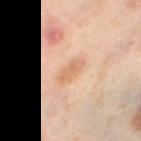Impression: Recorded during total-body skin imaging; not selected for excision or biopsy. Acquisition and patient details: Cropped from a whole-body photographic skin survey; the tile spans about 15 mm. On the right thigh. The recorded lesion diameter is about 3.5 mm. Automated tile analysis of the lesion measured a footprint of about 4 mm², a shape eccentricity near 0.9, and two-axis asymmetry of about 0.25. The software also gave a lesion color around L≈65 a*≈21 b*≈34 in CIELAB and a lesion-to-skin contrast of about 5.5 (normalized; higher = more distinct). It also reported a classifier nevus-likeness of about 10/100 and lesion-presence confidence of about 100/100. The tile uses cross-polarized illumination. The patient is a female about 50 years old.